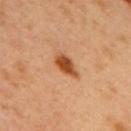Notes:
* workup: catalogued during a skin exam; not biopsied
* acquisition: ~15 mm crop, total-body skin-cancer survey
* subject: male, aged 48 to 52
* lighting: cross-polarized illumination
* automated lesion analysis: a lesion–skin lightness drop of about 15 and a lesion-to-skin contrast of about 10.5 (normalized; higher = more distinct); border irregularity of about 2 on a 0–10 scale, internal color variation of about 2.5 on a 0–10 scale, and peripheral color asymmetry of about 0.5
* anatomic site: the upper back